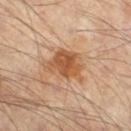A 15 mm close-up extracted from a 3D total-body photography capture. A male subject, aged 63–67. Approximately 5.5 mm at its widest. From the leg. Automated image analysis of the tile measured an area of roughly 13 mm², an outline eccentricity of about 0.75 (0 = round, 1 = elongated), and two-axis asymmetry of about 0.3. The software also gave a classifier nevus-likeness of about 90/100 and a detector confidence of about 100 out of 100 that the crop contains a lesion. Imaged with cross-polarized lighting.